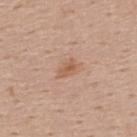{
  "biopsy_status": "not biopsied; imaged during a skin examination",
  "patient": {
    "sex": "male",
    "age_approx": 55
  },
  "site": "back",
  "lesion_size": {
    "long_diameter_mm_approx": 3.0
  },
  "image": {
    "source": "total-body photography crop",
    "field_of_view_mm": 15
  },
  "lighting": "white-light"
}